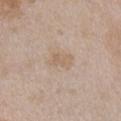Case summary:
– subject: female, about 30 years old
– image source: ~15 mm tile from a whole-body skin photo
– size: ~3 mm (longest diameter)
– automated metrics: a lesion–skin lightness drop of about 6; border irregularity of about 3 on a 0–10 scale, a color-variation rating of about 1.5/10, and peripheral color asymmetry of about 0.5
– site: the chest
– lighting: white-light